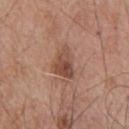The lesion was photographed on a routine skin check and not biopsied; there is no pathology result. The subject is a male aged 63–67. The lesion is located on the back. This is a white-light tile. A roughly 15 mm field-of-view crop from a total-body skin photograph. The lesion-visualizer software estimated internal color variation of about 6 on a 0–10 scale and a peripheral color-asymmetry measure near 2.